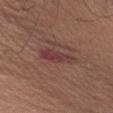Impression:
Part of a total-body skin-imaging series; this lesion was reviewed on a skin check and was not flagged for biopsy.
Clinical summary:
Approximately 5 mm at its widest. A 15 mm close-up extracted from a 3D total-body photography capture. A male subject aged 33 to 37. This is a white-light tile. Located on the left upper arm.